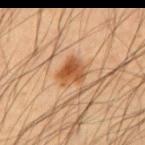Image and clinical context:
Cropped from a total-body skin-imaging series; the visible field is about 15 mm. The patient is a male in their mid- to late 50s. An algorithmic analysis of the crop reported border irregularity of about 2.5 on a 0–10 scale, internal color variation of about 5.5 on a 0–10 scale, and a peripheral color-asymmetry measure near 2. The analysis additionally found an automated nevus-likeness rating near 95 out of 100 and a lesion-detection confidence of about 100/100. The tile uses cross-polarized illumination. About 3 mm across. The lesion is on the abdomen.